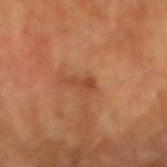This lesion was catalogued during total-body skin photography and was not selected for biopsy. A male patient, in their mid-70s. The lesion is on the right upper arm. This is a cross-polarized tile. Cropped from a total-body skin-imaging series; the visible field is about 15 mm. The lesion's longest dimension is about 3 mm.